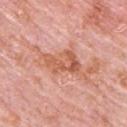{"patient": {"sex": "male", "age_approx": 80}, "automated_metrics": {"cielab_L": 59, "cielab_a": 28, "cielab_b": 34, "vs_skin_darker_L": 10.0, "nevus_likeness_0_100": 0}, "image": {"source": "total-body photography crop", "field_of_view_mm": 15}, "site": "upper back", "lighting": "white-light"}